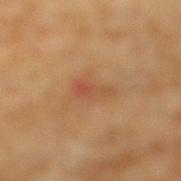Q: Was a biopsy performed?
A: total-body-photography surveillance lesion; no biopsy
Q: Who is the patient?
A: male, about 60 years old
Q: What did automated image analysis measure?
A: a footprint of about 3.5 mm², an eccentricity of roughly 0.85, and a symmetry-axis asymmetry near 0.5; a lesion color around L≈47 a*≈24 b*≈32 in CIELAB, roughly 7 lightness units darker than nearby skin, and a lesion-to-skin contrast of about 5.5 (normalized; higher = more distinct); a border-irregularity index near 5/10, internal color variation of about 1.5 on a 0–10 scale, and peripheral color asymmetry of about 0.5
Q: Lesion location?
A: the right lower leg
Q: What is the lesion's diameter?
A: ≈3 mm
Q: What is the imaging modality?
A: 15 mm crop, total-body photography